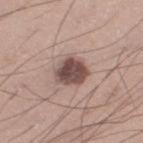Captured during whole-body skin photography for melanoma surveillance; the lesion was not biopsied. A 15 mm close-up extracted from a 3D total-body photography capture. On the leg. About 4 mm across. The patient is a male about 35 years old. Automated image analysis of the tile measured an average lesion color of about L≈49 a*≈18 b*≈21 (CIELAB), a lesion–skin lightness drop of about 16, and a lesion-to-skin contrast of about 11 (normalized; higher = more distinct). The software also gave a border-irregularity index near 2/10. The analysis additionally found a classifier nevus-likeness of about 65/100 and lesion-presence confidence of about 100/100. Imaged with white-light lighting.